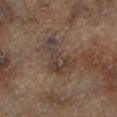Captured during whole-body skin photography for melanoma surveillance; the lesion was not biopsied. A male subject, aged approximately 65. A region of skin cropped from a whole-body photographic capture, roughly 15 mm wide. The lesion is located on the leg.